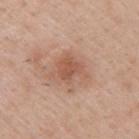The lesion was photographed on a routine skin check and not biopsied; there is no pathology result. On the right upper arm. A male patient aged 38–42. Cropped from a whole-body photographic skin survey; the tile spans about 15 mm.